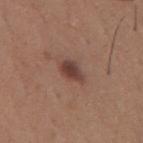biopsy status: catalogued during a skin exam; not biopsied
location: the mid back
imaging modality: ~15 mm crop, total-body skin-cancer survey
subject: male, in their mid- to late 60s